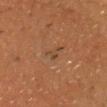Assessment:
Recorded during total-body skin imaging; not selected for excision or biopsy.
Background:
From the chest. Approximately 2.5 mm at its widest. A male patient, roughly 75 years of age. A lesion tile, about 15 mm wide, cut from a 3D total-body photograph.